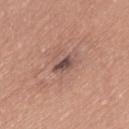{"patient": {"sex": "female", "age_approx": 45}, "lesion_size": {"long_diameter_mm_approx": 3.0}, "lighting": "white-light", "image": {"source": "total-body photography crop", "field_of_view_mm": 15}, "automated_metrics": {"color_variation_0_10": 4.0, "peripheral_color_asymmetry": 1.5}, "site": "leg"}